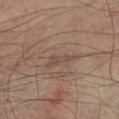Clinical impression: This lesion was catalogued during total-body skin photography and was not selected for biopsy.